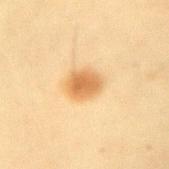notes=imaged on a skin check; not biopsied
location=the arm
lesion size=~3.5 mm (longest diameter)
image=15 mm crop, total-body photography
tile lighting=cross-polarized
subject=female, in their mid- to late 50s